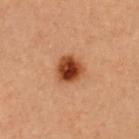Assessment: This lesion was catalogued during total-body skin photography and was not selected for biopsy. Clinical summary: Cropped from a whole-body photographic skin survey; the tile spans about 15 mm. The lesion is on the chest. This is a cross-polarized tile. A female subject aged 38 to 42.